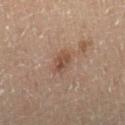Approximately 2.5 mm at its widest.
Captured under cross-polarized illumination.
A roughly 15 mm field-of-view crop from a total-body skin photograph.
From the right lower leg.
The patient is a male aged 68–72.